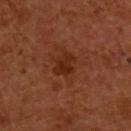A 15 mm close-up tile from a total-body photography series done for melanoma screening. A male subject in their mid-50s. The tile uses cross-polarized illumination. Approximately 3 mm at its widest. The lesion is on the upper back.